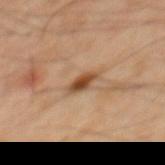biopsy status: total-body-photography surveillance lesion; no biopsy | imaging modality: ~15 mm crop, total-body skin-cancer survey | anatomic site: the mid back | tile lighting: cross-polarized | subject: male, aged around 45.